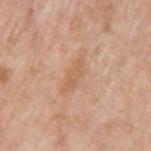Clinical impression:
The lesion was tiled from a total-body skin photograph and was not biopsied.
Acquisition and patient details:
A region of skin cropped from a whole-body photographic capture, roughly 15 mm wide. The lesion is on the arm. A male subject aged approximately 70. Longest diameter approximately 5.5 mm. The tile uses white-light illumination.